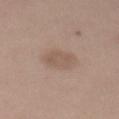  biopsy_status: not biopsied; imaged during a skin examination
  site: mid back
  lesion_size:
    long_diameter_mm_approx: 4.0
  image:
    source: total-body photography crop
    field_of_view_mm: 15
  patient:
    sex: female
    age_approx: 30
  automated_metrics:
    area_mm2_approx: 8.0
    eccentricity: 0.7
    shape_asymmetry: 0.2
    cielab_L: 55
    cielab_a: 16
    cielab_b: 25
    vs_skin_darker_L: 7.0
    vs_skin_contrast_norm: 5.5